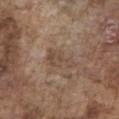Assessment: Recorded during total-body skin imaging; not selected for excision or biopsy. Acquisition and patient details: The patient is a male approximately 75 years of age. Captured under white-light illumination. A 15 mm crop from a total-body photograph taken for skin-cancer surveillance. From the chest.